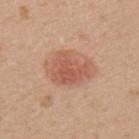Captured during whole-body skin photography for melanoma surveillance; the lesion was not biopsied. Imaged with white-light lighting. Automated image analysis of the tile measured two-axis asymmetry of about 0.35. It also reported an average lesion color of about L≈56 a*≈25 b*≈31 (CIELAB), roughly 10 lightness units darker than nearby skin, and a normalized lesion–skin contrast near 6.5. It also reported a border-irregularity rating of about 3.5/10, a color-variation rating of about 3/10, and peripheral color asymmetry of about 1. On the upper back. A close-up tile cropped from a whole-body skin photograph, about 15 mm across. A female subject roughly 70 years of age. The recorded lesion diameter is about 4.5 mm.